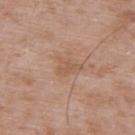* follow-up: catalogued during a skin exam; not biopsied
* location: the upper back
* subject: male, aged 48 to 52
* imaging modality: ~15 mm crop, total-body skin-cancer survey
* tile lighting: white-light